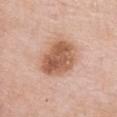No biopsy was performed on this lesion — it was imaged during a full skin examination and was not determined to be concerning. The lesion's longest dimension is about 5.5 mm. The lesion is located on the front of the torso. Automated image analysis of the tile measured a lesion area of about 17 mm², an outline eccentricity of about 0.65 (0 = round, 1 = elongated), and two-axis asymmetry of about 0.15. And it measured about 14 CIELAB-L* units darker than the surrounding skin and a normalized border contrast of about 9. Imaged with white-light lighting. A female patient roughly 75 years of age. A roughly 15 mm field-of-view crop from a total-body skin photograph.